The lesion is located on the front of the torso · a male subject, aged 43 to 47 · captured under cross-polarized illumination · measured at roughly 7.5 mm in maximum diameter · a lesion tile, about 15 mm wide, cut from a 3D total-body photograph — 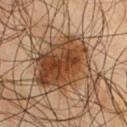histopathology: a melanoma in situ (malignant).